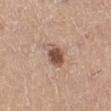biopsy status — catalogued during a skin exam; not biopsied | illumination — white-light illumination | anatomic site — the left lower leg | image source — total-body-photography crop, ~15 mm field of view | patient — female, approximately 60 years of age.